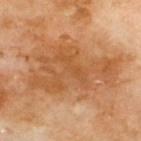{
  "biopsy_status": "not biopsied; imaged during a skin examination",
  "patient": {
    "sex": "male",
    "age_approx": 70
  },
  "site": "upper back",
  "image": {
    "source": "total-body photography crop",
    "field_of_view_mm": 15
  }
}